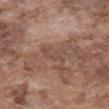{"lighting": "white-light", "image": {"source": "total-body photography crop", "field_of_view_mm": 15}, "site": "abdomen", "patient": {"sex": "male", "age_approx": 75}}